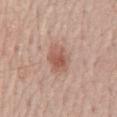Q: Was a biopsy performed?
A: no biopsy performed (imaged during a skin exam)
Q: Who is the patient?
A: male, approximately 70 years of age
Q: What is the imaging modality?
A: total-body-photography crop, ~15 mm field of view
Q: Illumination type?
A: white-light
Q: Lesion location?
A: the lower back
Q: How large is the lesion?
A: ≈3.5 mm